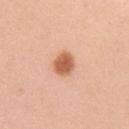| feature | finding |
|---|---|
| acquisition | 15 mm crop, total-body photography |
| body site | the left upper arm |
| subject | female, about 25 years old |